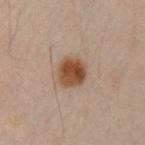The lesion was tiled from a total-body skin photograph and was not biopsied. This image is a 15 mm lesion crop taken from a total-body photograph. The tile uses cross-polarized illumination. The lesion is on the left upper arm. Approximately 4 mm at its widest. A male patient roughly 50 years of age.